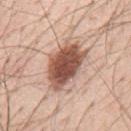Notes:
• follow-up — no biopsy performed (imaged during a skin exam)
• size — about 6 mm
• anatomic site — the mid back
• lighting — white-light
• image — ~15 mm tile from a whole-body skin photo
• patient — male, aged 53 to 57
• automated metrics — an eccentricity of roughly 0.8 and a shape-asymmetry score of about 0.2 (0 = symmetric); an average lesion color of about L≈54 a*≈22 b*≈27 (CIELAB), roughly 19 lightness units darker than nearby skin, and a lesion-to-skin contrast of about 11.5 (normalized; higher = more distinct); a color-variation rating of about 5.5/10 and a peripheral color-asymmetry measure near 1.5